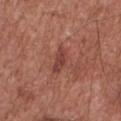notes: imaged on a skin check; not biopsied
TBP lesion metrics: a mean CIELAB color near L≈42 a*≈27 b*≈26, a lesion–skin lightness drop of about 8, and a lesion-to-skin contrast of about 6.5 (normalized; higher = more distinct); a border-irregularity index near 3/10, internal color variation of about 2.5 on a 0–10 scale, and a peripheral color-asymmetry measure near 1
patient: male, about 75 years old
size: ≈3 mm
acquisition: ~15 mm crop, total-body skin-cancer survey
location: the chest
illumination: white-light